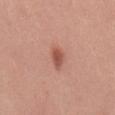| feature | finding |
|---|---|
| follow-up | catalogued during a skin exam; not biopsied |
| image | ~15 mm crop, total-body skin-cancer survey |
| TBP lesion metrics | a footprint of about 4 mm², an outline eccentricity of about 0.8 (0 = round, 1 = elongated), and a shape-asymmetry score of about 0.3 (0 = symmetric); a border-irregularity rating of about 2.5/10, a within-lesion color-variation index near 2.5/10, and radial color variation of about 1; a detector confidence of about 100 out of 100 that the crop contains a lesion |
| patient | female, aged 23 to 27 |
| lesion size | ≈3 mm |
| body site | the mid back |